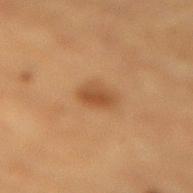{
  "biopsy_status": "not biopsied; imaged during a skin examination",
  "lesion_size": {
    "long_diameter_mm_approx": 3.0
  },
  "lighting": "cross-polarized",
  "image": {
    "source": "total-body photography crop",
    "field_of_view_mm": 15
  },
  "patient": {
    "sex": "male",
    "age_approx": "85+"
  },
  "site": "lower back"
}